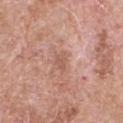Clinical impression:
Recorded during total-body skin imaging; not selected for excision or biopsy.
Clinical summary:
Longest diameter approximately 2.5 mm. The patient is a male aged approximately 65. A lesion tile, about 15 mm wide, cut from a 3D total-body photograph. The tile uses white-light illumination. The lesion is on the right upper arm.